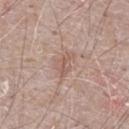No biopsy was performed on this lesion — it was imaged during a full skin examination and was not determined to be concerning.
A male patient, aged 63 to 67.
Cropped from a total-body skin-imaging series; the visible field is about 15 mm.
Located on the abdomen.
This is a white-light tile.
An algorithmic analysis of the crop reported a lesion color around L≈57 a*≈19 b*≈25 in CIELAB, roughly 7 lightness units darker than nearby skin, and a lesion-to-skin contrast of about 5 (normalized; higher = more distinct). It also reported a lesion-detection confidence of about 100/100.